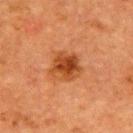Captured under cross-polarized illumination.
A female subject, roughly 55 years of age.
A region of skin cropped from a whole-body photographic capture, roughly 15 mm wide.
Located on the upper back.